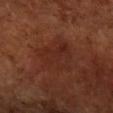Clinical impression: The lesion was tiled from a total-body skin photograph and was not biopsied. Acquisition and patient details: The lesion is on the right forearm. Approximately 5 mm at its widest. A female subject roughly 65 years of age. A region of skin cropped from a whole-body photographic capture, roughly 15 mm wide. Imaged with cross-polarized lighting.